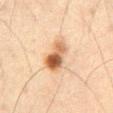Q: Was a biopsy performed?
A: total-body-photography surveillance lesion; no biopsy
Q: What did automated image analysis measure?
A: a border-irregularity rating of about 2.5/10
Q: Who is the patient?
A: male, aged 63–67
Q: What is the anatomic site?
A: the lower back
Q: How was this image acquired?
A: total-body-photography crop, ~15 mm field of view